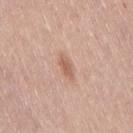Notes:
• notes: total-body-photography surveillance lesion; no biopsy
• TBP lesion metrics: an area of roughly 4 mm² and an outline eccentricity of about 0.9 (0 = round, 1 = elongated); a border-irregularity index near 3.5/10, internal color variation of about 1.5 on a 0–10 scale, and radial color variation of about 0.5; lesion-presence confidence of about 100/100
• subject: female, about 40 years old
• image source: total-body-photography crop, ~15 mm field of view
• lesion diameter: ~3.5 mm (longest diameter)
• location: the lower back
• tile lighting: white-light illumination